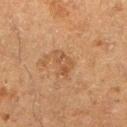Clinical impression:
Part of a total-body skin-imaging series; this lesion was reviewed on a skin check and was not flagged for biopsy.
Clinical summary:
Longest diameter approximately 3.5 mm. The lesion is on the left lower leg. A female patient, aged approximately 50. The lesion-visualizer software estimated an average lesion color of about L≈43 a*≈18 b*≈30 (CIELAB), about 6 CIELAB-L* units darker than the surrounding skin, and a lesion-to-skin contrast of about 5 (normalized; higher = more distinct). The software also gave a border-irregularity index near 3.5/10, a color-variation rating of about 3.5/10, and a peripheral color-asymmetry measure near 1. It also reported a nevus-likeness score of about 0/100 and lesion-presence confidence of about 100/100. A lesion tile, about 15 mm wide, cut from a 3D total-body photograph.